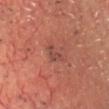Q: Was a biopsy performed?
A: total-body-photography surveillance lesion; no biopsy
Q: What is the anatomic site?
A: the chest
Q: What is the imaging modality?
A: ~15 mm crop, total-body skin-cancer survey
Q: What lighting was used for the tile?
A: cross-polarized
Q: Automated lesion metrics?
A: an area of roughly 3 mm², an eccentricity of roughly 0.8, and two-axis asymmetry of about 0.55; lesion-presence confidence of about 100/100
Q: Who is the patient?
A: male, aged 63–67